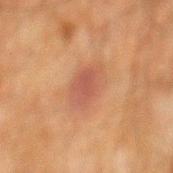This lesion was catalogued during total-body skin photography and was not selected for biopsy. A male subject, about 60 years old. The lesion is on the mid back. A roughly 15 mm field-of-view crop from a total-body skin photograph. Automated image analysis of the tile measured a footprint of about 11 mm² and a shape-asymmetry score of about 0.15 (0 = symmetric). This is a cross-polarized tile. Longest diameter approximately 4.5 mm.